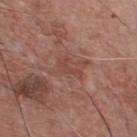Imaged during a routine full-body skin examination; the lesion was not biopsied and no histopathology is available. The lesion is located on the chest. The patient is a male aged 73–77. This is a white-light tile. Cropped from a total-body skin-imaging series; the visible field is about 15 mm.